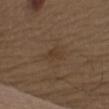Impression:
Part of a total-body skin-imaging series; this lesion was reviewed on a skin check and was not flagged for biopsy.
Acquisition and patient details:
Captured under white-light illumination. An algorithmic analysis of the crop reported a lesion color around L≈37 a*≈15 b*≈28 in CIELAB, roughly 5 lightness units darker than nearby skin, and a lesion-to-skin contrast of about 5 (normalized; higher = more distinct). The software also gave a nevus-likeness score of about 0/100 and lesion-presence confidence of about 100/100. Located on the upper back. This image is a 15 mm lesion crop taken from a total-body photograph. A female subject approximately 40 years of age. The lesion's longest dimension is about 3.5 mm.